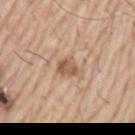Recorded during total-body skin imaging; not selected for excision or biopsy. The lesion is located on the left upper arm. The tile uses white-light illumination. A male patient roughly 55 years of age. Cropped from a total-body skin-imaging series; the visible field is about 15 mm.